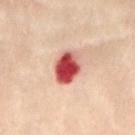Assessment:
This lesion was catalogued during total-body skin photography and was not selected for biopsy.